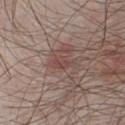Clinical impression: Imaged during a routine full-body skin examination; the lesion was not biopsied and no histopathology is available. Acquisition and patient details: A region of skin cropped from a whole-body photographic capture, roughly 15 mm wide. The tile uses white-light illumination. A male patient aged 28 to 32. Automated tile analysis of the lesion measured a footprint of about 4.5 mm² and a shape eccentricity near 0.9. The software also gave a lesion color around L≈46 a*≈19 b*≈21 in CIELAB and a lesion-to-skin contrast of about 6 (normalized; higher = more distinct). The software also gave an automated nevus-likeness rating near 45 out of 100 and a lesion-detection confidence of about 100/100. Measured at roughly 3.5 mm in maximum diameter.